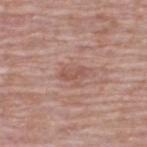Q: Was a biopsy performed?
A: imaged on a skin check; not biopsied
Q: What kind of image is this?
A: total-body-photography crop, ~15 mm field of view
Q: Illumination type?
A: white-light illumination
Q: Where on the body is the lesion?
A: the upper back
Q: What did automated image analysis measure?
A: a lesion area of about 3 mm² and a symmetry-axis asymmetry near 0.3; a normalized border contrast of about 5.5; a border-irregularity index near 3.5/10, a color-variation rating of about 0/10, and peripheral color asymmetry of about 0; an automated nevus-likeness rating near 0 out of 100 and lesion-presence confidence of about 100/100
Q: Patient demographics?
A: male, approximately 65 years of age